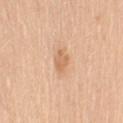Captured during whole-body skin photography for melanoma surveillance; the lesion was not biopsied.
Located on the right upper arm.
About 2.5 mm across.
A female subject aged around 55.
A region of skin cropped from a whole-body photographic capture, roughly 15 mm wide.